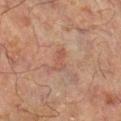Imaged during a routine full-body skin examination; the lesion was not biopsied and no histopathology is available. Captured under cross-polarized illumination. A region of skin cropped from a whole-body photographic capture, roughly 15 mm wide. The lesion's longest dimension is about 3 mm. The patient is a male about 65 years old. From the left lower leg. Automated image analysis of the tile measured a lesion area of about 3.5 mm², an eccentricity of roughly 0.85, and a symmetry-axis asymmetry near 0.4. The software also gave a border-irregularity index near 4/10, a color-variation rating of about 1/10, and radial color variation of about 0. The software also gave a lesion-detection confidence of about 100/100.